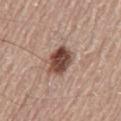About 4 mm across. The subject is a male roughly 60 years of age. The lesion is located on the mid back. A roughly 15 mm field-of-view crop from a total-body skin photograph.